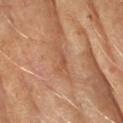<case>
<biopsy_status>not biopsied; imaged during a skin examination</biopsy_status>
<patient>
  <sex>female</sex>
  <age_approx>60</age_approx>
</patient>
<site>right forearm</site>
<image>
  <source>total-body photography crop</source>
  <field_of_view_mm>15</field_of_view_mm>
</image>
</case>